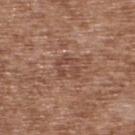No biopsy was performed on this lesion — it was imaged during a full skin examination and was not determined to be concerning. The lesion is on the upper back. Cropped from a whole-body photographic skin survey; the tile spans about 15 mm. Automated tile analysis of the lesion measured an average lesion color of about L≈46 a*≈21 b*≈27 (CIELAB), about 7 CIELAB-L* units darker than the surrounding skin, and a normalized border contrast of about 5.5. It also reported a nevus-likeness score of about 0/100. The subject is a male aged approximately 75. Measured at roughly 3 mm in maximum diameter. This is a white-light tile.